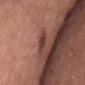No biopsy was performed on this lesion — it was imaged during a full skin examination and was not determined to be concerning. Captured under white-light illumination. Cropped from a whole-body photographic skin survey; the tile spans about 15 mm. Automated tile analysis of the lesion measured an area of roughly 3 mm². The software also gave a lesion color around L≈40 a*≈22 b*≈22 in CIELAB, about 9 CIELAB-L* units darker than the surrounding skin, and a normalized border contrast of about 7.5. The software also gave a classifier nevus-likeness of about 0/100 and a lesion-detection confidence of about 95/100. The subject is a male about 40 years old. From the abdomen. Approximately 3 mm at its widest.